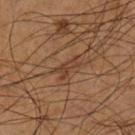Clinical impression: Imaged during a routine full-body skin examination; the lesion was not biopsied and no histopathology is available. Clinical summary: A 15 mm close-up tile from a total-body photography series done for melanoma screening. A male patient aged approximately 55. The lesion is on the left lower leg.